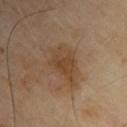Findings:
• follow-up · total-body-photography surveillance lesion; no biopsy
• image-analysis metrics · a lesion area of about 13 mm², an eccentricity of roughly 0.8, and a shape-asymmetry score of about 0.4 (0 = symmetric); a mean CIELAB color near L≈44 a*≈16 b*≈31, roughly 8 lightness units darker than nearby skin, and a normalized border contrast of about 7; internal color variation of about 3.5 on a 0–10 scale and radial color variation of about 1
• image · ~15 mm tile from a whole-body skin photo
• subject · male, roughly 65 years of age
• illumination · cross-polarized
• anatomic site · the right upper arm
• size · ~6 mm (longest diameter)